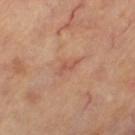biopsy status = total-body-photography surveillance lesion; no biopsy | acquisition = ~15 mm crop, total-body skin-cancer survey | tile lighting = cross-polarized illumination | site = the right leg | patient = female, aged 58 to 62.